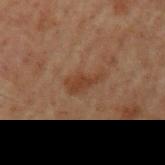Q: Is there a histopathology result?
A: total-body-photography surveillance lesion; no biopsy
Q: How large is the lesion?
A: ~4 mm (longest diameter)
Q: Where on the body is the lesion?
A: the left upper arm
Q: What did automated image analysis measure?
A: border irregularity of about 5 on a 0–10 scale, a within-lesion color-variation index near 2/10, and radial color variation of about 0.5; a lesion-detection confidence of about 100/100
Q: Patient demographics?
A: male, roughly 60 years of age
Q: How was this image acquired?
A: ~15 mm tile from a whole-body skin photo
Q: How was the tile lit?
A: cross-polarized